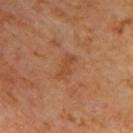follow-up: catalogued during a skin exam; not biopsied | patient: male, in their 60s | diameter: ~3 mm (longest diameter) | imaging modality: ~15 mm crop, total-body skin-cancer survey | TBP lesion metrics: a footprint of about 3.5 mm², an outline eccentricity of about 0.9 (0 = round, 1 = elongated), and two-axis asymmetry of about 0.4; a lesion color around L≈45 a*≈24 b*≈35 in CIELAB and a normalized border contrast of about 5.5 | location: the upper back | illumination: cross-polarized illumination.